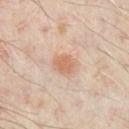The lesion was tiled from a total-body skin photograph and was not biopsied. Located on the chest. A male patient roughly 50 years of age. Approximately 2.5 mm at its widest. Imaged with cross-polarized lighting. Cropped from a whole-body photographic skin survey; the tile spans about 15 mm.